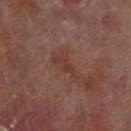The lesion was tiled from a total-body skin photograph and was not biopsied.
The patient is a male in their 70s.
On the leg.
Cropped from a whole-body photographic skin survey; the tile spans about 15 mm.
The total-body-photography lesion software estimated a border-irregularity rating of about 5/10 and peripheral color asymmetry of about 0.5. And it measured a lesion-detection confidence of about 100/100.
Captured under cross-polarized illumination.